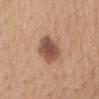Assessment: The lesion was tiled from a total-body skin photograph and was not biopsied. Background: Located on the mid back. The lesion's longest dimension is about 4 mm. Automated image analysis of the tile measured a mean CIELAB color near L≈51 a*≈20 b*≈28, a lesion–skin lightness drop of about 14, and a normalized lesion–skin contrast near 10. And it measured a within-lesion color-variation index near 4/10 and peripheral color asymmetry of about 1. And it measured a classifier nevus-likeness of about 80/100 and a lesion-detection confidence of about 100/100. The subject is a female in their mid- to late 60s. A lesion tile, about 15 mm wide, cut from a 3D total-body photograph. Captured under white-light illumination.